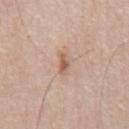biopsy_status: not biopsied; imaged during a skin examination
patient:
  sex: male
  age_approx: 55
image:
  source: total-body photography crop
  field_of_view_mm: 15
site: chest
lesion_size:
  long_diameter_mm_approx: 2.5
lighting: white-light